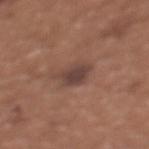The lesion was tiled from a total-body skin photograph and was not biopsied.
On the chest.
The tile uses white-light illumination.
Longest diameter approximately 4 mm.
A 15 mm close-up extracted from a 3D total-body photography capture.
The patient is a female aged around 35.